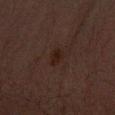Impression: Recorded during total-body skin imaging; not selected for excision or biopsy. Clinical summary: On the right forearm. The recorded lesion diameter is about 2.5 mm. A close-up tile cropped from a whole-body skin photograph, about 15 mm across. Captured under cross-polarized illumination. An algorithmic analysis of the crop reported a lesion area of about 3.5 mm² and an eccentricity of roughly 0.75. The analysis additionally found a border-irregularity rating of about 2/10 and peripheral color asymmetry of about 0.5. A male subject aged around 30.